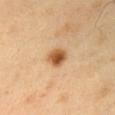Impression:
Imaged during a routine full-body skin examination; the lesion was not biopsied and no histopathology is available.
Context:
The tile uses cross-polarized illumination. A roughly 15 mm field-of-view crop from a total-body skin photograph. The lesion-visualizer software estimated roughly 15 lightness units darker than nearby skin and a normalized lesion–skin contrast near 10. On the chest. A male subject, approximately 50 years of age.